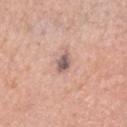Q: Was a biopsy performed?
A: catalogued during a skin exam; not biopsied
Q: What lighting was used for the tile?
A: white-light
Q: Lesion location?
A: the head or neck
Q: What is the lesion's diameter?
A: ≈3 mm
Q: What kind of image is this?
A: ~15 mm tile from a whole-body skin photo
Q: What are the patient's age and sex?
A: female, aged 43 to 47